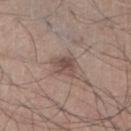Acquisition and patient details: This image is a 15 mm lesion crop taken from a total-body photograph. A male patient aged 63–67. On the right lower leg.